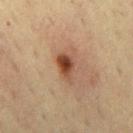biopsy_status: not biopsied; imaged during a skin examination
patient:
  sex: male
  age_approx: 65
image:
  source: total-body photography crop
  field_of_view_mm: 15
site: mid back
lighting: cross-polarized